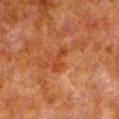Assessment:
Imaged during a routine full-body skin examination; the lesion was not biopsied and no histopathology is available.
Acquisition and patient details:
The patient is a male about 80 years old. Approximately 3 mm at its widest. A region of skin cropped from a whole-body photographic capture, roughly 15 mm wide. This is a cross-polarized tile. Located on the left lower leg.